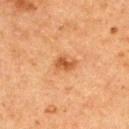The recorded lesion diameter is about 2.5 mm. A 15 mm crop from a total-body photograph taken for skin-cancer surveillance. A female subject approximately 60 years of age. Automated tile analysis of the lesion measured an average lesion color of about L≈45 a*≈24 b*≈36 (CIELAB), roughly 10 lightness units darker than nearby skin, and a normalized border contrast of about 8. On the back. Captured under cross-polarized illumination.